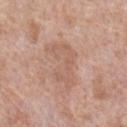Assessment:
No biopsy was performed on this lesion — it was imaged during a full skin examination and was not determined to be concerning.
Clinical summary:
Cropped from a whole-body photographic skin survey; the tile spans about 15 mm. Captured under white-light illumination. The lesion is located on the chest. A male subject aged around 60. About 6 mm across.